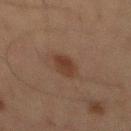Q: Was a biopsy performed?
A: catalogued during a skin exam; not biopsied
Q: What is the anatomic site?
A: the abdomen
Q: What did automated image analysis measure?
A: a lesion area of about 6 mm²; a lesion color around L≈29 a*≈16 b*≈22 in CIELAB, about 7 CIELAB-L* units darker than the surrounding skin, and a normalized lesion–skin contrast near 7.5; border irregularity of about 2 on a 0–10 scale and a peripheral color-asymmetry measure near 1; a nevus-likeness score of about 90/100 and a detector confidence of about 100 out of 100 that the crop contains a lesion
Q: How was this image acquired?
A: total-body-photography crop, ~15 mm field of view
Q: Illumination type?
A: cross-polarized
Q: What are the patient's age and sex?
A: male, in their mid- to late 60s
Q: How large is the lesion?
A: ≈3.5 mm